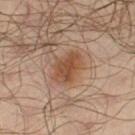notes: total-body-photography surveillance lesion; no biopsy
diameter: ~4 mm (longest diameter)
TBP lesion metrics: an area of roughly 8 mm², an eccentricity of roughly 0.75, and two-axis asymmetry of about 0.3; a lesion color around L≈45 a*≈20 b*≈30 in CIELAB and a lesion-to-skin contrast of about 8 (normalized; higher = more distinct)
subject: male, in their mid-60s
lighting: cross-polarized illumination
imaging modality: ~15 mm tile from a whole-body skin photo
location: the left thigh